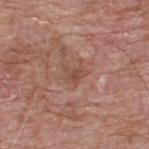Captured during whole-body skin photography for melanoma surveillance; the lesion was not biopsied. A lesion tile, about 15 mm wide, cut from a 3D total-body photograph. Imaged with white-light lighting. Approximately 2.5 mm at its widest. A male subject, aged 58 to 62. From the upper back.